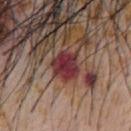Recorded during total-body skin imaging; not selected for excision or biopsy. A male subject, aged approximately 55. A 15 mm close-up extracted from a 3D total-body photography capture. On the chest.